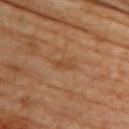Impression:
The lesion was tiled from a total-body skin photograph and was not biopsied.
Background:
About 3 mm across. The lesion is located on the back. This is a cross-polarized tile. Cropped from a whole-body photographic skin survey; the tile spans about 15 mm. A female patient, aged 63–67. The total-body-photography lesion software estimated a lesion–skin lightness drop of about 6 and a normalized border contrast of about 5.5.